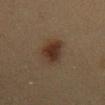patient: female, aged around 50; illumination: cross-polarized illumination; image source: ~15 mm crop, total-body skin-cancer survey; lesion diameter: ~4 mm (longest diameter); site: the mid back.